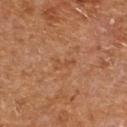Impression: Captured during whole-body skin photography for melanoma surveillance; the lesion was not biopsied.